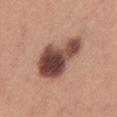Recorded during total-body skin imaging; not selected for excision or biopsy. A roughly 15 mm field-of-view crop from a total-body skin photograph. A female subject, in their mid-20s. This is a white-light tile. From the left thigh. The lesion-visualizer software estimated an area of roughly 22 mm², an outline eccentricity of about 0.9 (0 = round, 1 = elongated), and a symmetry-axis asymmetry near 0.35. And it measured a mean CIELAB color near L≈47 a*≈22 b*≈24, roughly 18 lightness units darker than nearby skin, and a lesion-to-skin contrast of about 13 (normalized; higher = more distinct). The software also gave a nevus-likeness score of about 20/100. Approximately 8.5 mm at its widest.